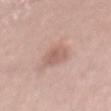Q: Automated lesion metrics?
A: an outline eccentricity of about 0.75 (0 = round, 1 = elongated) and a symmetry-axis asymmetry near 0.2; a lesion–skin lightness drop of about 9 and a lesion-to-skin contrast of about 6 (normalized; higher = more distinct); a color-variation rating of about 2/10; an automated nevus-likeness rating near 15 out of 100 and lesion-presence confidence of about 100/100
Q: Patient demographics?
A: male, in their mid-50s
Q: Lesion location?
A: the mid back
Q: What is the imaging modality?
A: ~15 mm crop, total-body skin-cancer survey